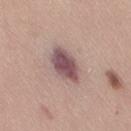The lesion was photographed on a routine skin check and not biopsied; there is no pathology result. This image is a 15 mm lesion crop taken from a total-body photograph. An algorithmic analysis of the crop reported a footprint of about 12 mm² and an outline eccentricity of about 0.7 (0 = round, 1 = elongated). The analysis additionally found an average lesion color of about L≈53 a*≈19 b*≈17 (CIELAB). And it measured a classifier nevus-likeness of about 50/100 and a detector confidence of about 100 out of 100 that the crop contains a lesion. The subject is a female aged 43 to 47. This is a white-light tile. From the right thigh.